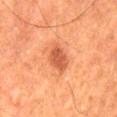Part of a total-body skin-imaging series; this lesion was reviewed on a skin check and was not flagged for biopsy.
A roughly 15 mm field-of-view crop from a total-body skin photograph.
The total-body-photography lesion software estimated a mean CIELAB color near L≈52 a*≈30 b*≈37 and a lesion-to-skin contrast of about 7.5 (normalized; higher = more distinct). The software also gave a classifier nevus-likeness of about 85/100 and a detector confidence of about 100 out of 100 that the crop contains a lesion.
A male subject about 65 years old.
The tile uses cross-polarized illumination.
On the left thigh.
Measured at roughly 3.5 mm in maximum diameter.